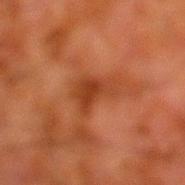Acquisition and patient details: The lesion is on the leg. A male subject, roughly 80 years of age. Approximately 5 mm at its widest. A roughly 15 mm field-of-view crop from a total-body skin photograph. Imaged with cross-polarized lighting.